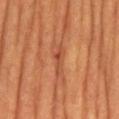illumination: cross-polarized
lesion size: ≈4 mm
anatomic site: the abdomen
subject: male, aged 63 to 67
image: total-body-photography crop, ~15 mm field of view
image-analysis metrics: a lesion area of about 5 mm², an eccentricity of roughly 0.95, and a shape-asymmetry score of about 0.35 (0 = symmetric); a lesion color around L≈46 a*≈26 b*≈34 in CIELAB, a lesion–skin lightness drop of about 8, and a normalized border contrast of about 6; a border-irregularity index near 4.5/10, internal color variation of about 3 on a 0–10 scale, and radial color variation of about 0.5